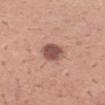notes = catalogued during a skin exam; not biopsied
subject = male, aged approximately 40
acquisition = ~15 mm tile from a whole-body skin photo
anatomic site = the right forearm